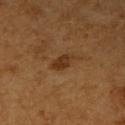Q: Was a biopsy performed?
A: total-body-photography surveillance lesion; no biopsy
Q: Patient demographics?
A: male, approximately 60 years of age
Q: How was this image acquired?
A: total-body-photography crop, ~15 mm field of view
Q: How was the tile lit?
A: cross-polarized illumination
Q: What is the lesion's diameter?
A: about 3.5 mm
Q: Lesion location?
A: the right upper arm